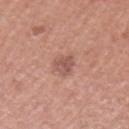Impression: Captured during whole-body skin photography for melanoma surveillance; the lesion was not biopsied. Image and clinical context: A roughly 15 mm field-of-view crop from a total-body skin photograph. Longest diameter approximately 3 mm. The lesion is on the left upper arm. A female subject, about 50 years old. Imaged with white-light lighting.